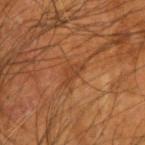Clinical impression:
This lesion was catalogued during total-body skin photography and was not selected for biopsy.
Image and clinical context:
The total-body-photography lesion software estimated a lesion area of about 3 mm² and an outline eccentricity of about 0.85 (0 = round, 1 = elongated). The software also gave a border-irregularity rating of about 5.5/10 and internal color variation of about 1.5 on a 0–10 scale. The software also gave an automated nevus-likeness rating near 0 out of 100 and a detector confidence of about 80 out of 100 that the crop contains a lesion. Imaged with cross-polarized lighting. A male patient in their mid- to late 50s. On the left forearm. Measured at roughly 3 mm in maximum diameter. Cropped from a total-body skin-imaging series; the visible field is about 15 mm.